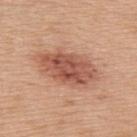This lesion was catalogued during total-body skin photography and was not selected for biopsy.
A male patient, about 70 years old.
The lesion is located on the upper back.
Imaged with white-light lighting.
A region of skin cropped from a whole-body photographic capture, roughly 15 mm wide.
Longest diameter approximately 7 mm.